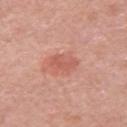follow-up: catalogued during a skin exam; not biopsied | patient: male, approximately 50 years of age | imaging modality: ~15 mm crop, total-body skin-cancer survey | tile lighting: white-light | automated metrics: an outline eccentricity of about 0.7 (0 = round, 1 = elongated) and a symmetry-axis asymmetry near 0.2; a border-irregularity rating of about 2/10, a within-lesion color-variation index near 2/10, and radial color variation of about 0.5 | body site: the left upper arm | lesion size: about 3 mm.